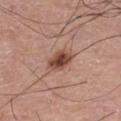A male subject, roughly 75 years of age. The recorded lesion diameter is about 3 mm. Automated tile analysis of the lesion measured a footprint of about 7 mm², an eccentricity of roughly 0.6, and two-axis asymmetry of about 0.15. A close-up tile cropped from a whole-body skin photograph, about 15 mm across. On the leg. The tile uses white-light illumination.